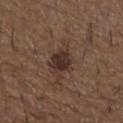Recorded during total-body skin imaging; not selected for excision or biopsy.